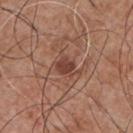The lesion was tiled from a total-body skin photograph and was not biopsied. Imaged with white-light lighting. The subject is a male about 55 years old. A 15 mm close-up extracted from a 3D total-body photography capture. The lesion is located on the chest.